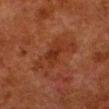| feature | finding |
|---|---|
| workup | imaged on a skin check; not biopsied |
| lesion diameter | about 6.5 mm |
| automated lesion analysis | an area of roughly 12 mm², an outline eccentricity of about 0.95 (0 = round, 1 = elongated), and two-axis asymmetry of about 0.4; a border-irregularity index near 5.5/10, internal color variation of about 3 on a 0–10 scale, and peripheral color asymmetry of about 1 |
| image source | ~15 mm crop, total-body skin-cancer survey |
| lighting | cross-polarized illumination |
| subject | male, roughly 80 years of age |
| site | the left lower leg |